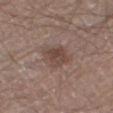Part of a total-body skin-imaging series; this lesion was reviewed on a skin check and was not flagged for biopsy.
A male patient, approximately 65 years of age.
Located on the right thigh.
Cropped from a total-body skin-imaging series; the visible field is about 15 mm.